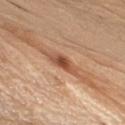Q: How was this image acquired?
A: ~15 mm tile from a whole-body skin photo
Q: What is the lesion's diameter?
A: ~4 mm (longest diameter)
Q: Lesion location?
A: the right upper arm
Q: How was the tile lit?
A: white-light
Q: Who is the patient?
A: male, roughly 70 years of age